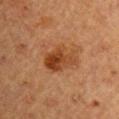Q: Was a biopsy performed?
A: catalogued during a skin exam; not biopsied
Q: Automated lesion metrics?
A: a lesion color around L≈36 a*≈22 b*≈33 in CIELAB, about 9 CIELAB-L* units darker than the surrounding skin, and a normalized border contrast of about 8.5; a nevus-likeness score of about 95/100
Q: Patient demographics?
A: female, aged 68 to 72
Q: Lesion location?
A: the right upper arm
Q: What kind of image is this?
A: total-body-photography crop, ~15 mm field of view
Q: Illumination type?
A: cross-polarized illumination
Q: How large is the lesion?
A: ≈4.5 mm